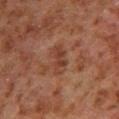<lesion>
<biopsy_status>not biopsied; imaged during a skin examination</biopsy_status>
<lesion_size>
  <long_diameter_mm_approx>4.0</long_diameter_mm_approx>
</lesion_size>
<image>
  <source>total-body photography crop</source>
  <field_of_view_mm>15</field_of_view_mm>
</image>
<site>right lower leg</site>
<lighting>cross-polarized</lighting>
<patient>
  <sex>male</sex>
  <age_approx>80</age_approx>
</patient>
</lesion>